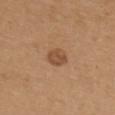This lesion was catalogued during total-body skin photography and was not selected for biopsy. A region of skin cropped from a whole-body photographic capture, roughly 15 mm wide. A female patient aged approximately 40. On the right upper arm. The lesion-visualizer software estimated an area of roughly 4.5 mm², an outline eccentricity of about 0.65 (0 = round, 1 = elongated), and two-axis asymmetry of about 0.15. The analysis additionally found roughly 10 lightness units darker than nearby skin and a normalized border contrast of about 7. It also reported border irregularity of about 1.5 on a 0–10 scale, internal color variation of about 2.5 on a 0–10 scale, and radial color variation of about 1. The software also gave a lesion-detection confidence of about 100/100.